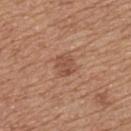• notes — imaged on a skin check; not biopsied
• acquisition — 15 mm crop, total-body photography
• lesion size — about 3 mm
• subject — female, in their mid- to late 50s
• TBP lesion metrics — a footprint of about 5.5 mm², a shape eccentricity near 0.5, and a symmetry-axis asymmetry near 0.15; an average lesion color of about L≈51 a*≈22 b*≈30 (CIELAB) and roughly 8 lightness units darker than nearby skin; a border-irregularity rating of about 2/10, a color-variation rating of about 2/10, and a peripheral color-asymmetry measure near 1
• body site — the back